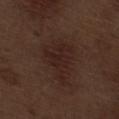Assessment:
This lesion was catalogued during total-body skin photography and was not selected for biopsy.
Background:
From the right thigh. This is a white-light tile. The subject is a male about 70 years old. Approximately 6 mm at its widest. Automated tile analysis of the lesion measured an area of roughly 16 mm². The software also gave a lesion color around L≈23 a*≈16 b*≈20 in CIELAB, about 5 CIELAB-L* units darker than the surrounding skin, and a normalized border contrast of about 6.5. The software also gave a detector confidence of about 100 out of 100 that the crop contains a lesion. A lesion tile, about 15 mm wide, cut from a 3D total-body photograph.